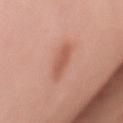Imaged during a routine full-body skin examination; the lesion was not biopsied and no histopathology is available. A female subject aged 48–52. From the chest. Imaged with white-light lighting. The recorded lesion diameter is about 3 mm. Cropped from a whole-body photographic skin survey; the tile spans about 15 mm.